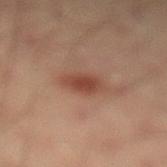biopsy_status: not biopsied; imaged during a skin examination
patient:
  sex: male
  age_approx: 40
site: left lower leg
lighting: cross-polarized
image:
  source: total-body photography crop
  field_of_view_mm: 15